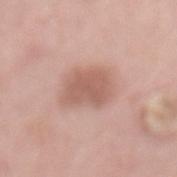{
  "biopsy_status": "not biopsied; imaged during a skin examination",
  "site": "front of the torso",
  "patient": {
    "sex": "female",
    "age_approx": 65
  },
  "lesion_size": {
    "long_diameter_mm_approx": 4.0
  },
  "image": {
    "source": "total-body photography crop",
    "field_of_view_mm": 15
  }
}